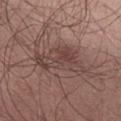Captured during whole-body skin photography for melanoma surveillance; the lesion was not biopsied.
Automated image analysis of the tile measured a lesion area of about 14 mm², an outline eccentricity of about 0.85 (0 = round, 1 = elongated), and a symmetry-axis asymmetry near 0.45. The analysis additionally found a lesion color around L≈43 a*≈18 b*≈21 in CIELAB, a lesion–skin lightness drop of about 8, and a lesion-to-skin contrast of about 6.5 (normalized; higher = more distinct). And it measured a border-irregularity index near 5.5/10 and radial color variation of about 1.5. And it measured lesion-presence confidence of about 95/100.
A close-up tile cropped from a whole-body skin photograph, about 15 mm across.
A male subject, in their mid- to late 60s.
Measured at roughly 6.5 mm in maximum diameter.
The lesion is located on the left thigh.